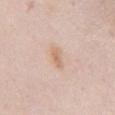Captured under white-light illumination.
The lesion is located on the chest.
The subject is a female aged around 40.
Cropped from a whole-body photographic skin survey; the tile spans about 15 mm.
Measured at roughly 2.5 mm in maximum diameter.